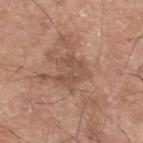  biopsy_status: not biopsied; imaged during a skin examination
  site: left lower leg
  lesion_size:
    long_diameter_mm_approx: 4.0
  image:
    source: total-body photography crop
    field_of_view_mm: 15
  automated_metrics:
    area_mm2_approx: 8.0
    eccentricity: 0.55
    shape_asymmetry: 0.4
    cielab_L: 51
    cielab_a: 20
    cielab_b: 28
    vs_skin_contrast_norm: 5.5
    border_irregularity_0_10: 6.5
    color_variation_0_10: 2.5
    peripheral_color_asymmetry: 1.0
    nevus_likeness_0_100: 0
    lesion_detection_confidence_0_100: 95
  patient:
    sex: male
    age_approx: 60